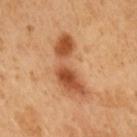Assessment: No biopsy was performed on this lesion — it was imaged during a full skin examination and was not determined to be concerning. Acquisition and patient details: A close-up tile cropped from a whole-body skin photograph, about 15 mm across. The subject is a male aged approximately 50. About 7.5 mm across. The lesion is on the right upper arm. An algorithmic analysis of the crop reported a shape eccentricity near 0.95 and a symmetry-axis asymmetry near 0.45. The software also gave an average lesion color of about L≈52 a*≈26 b*≈38 (CIELAB), roughly 13 lightness units darker than nearby skin, and a lesion-to-skin contrast of about 9 (normalized; higher = more distinct). Captured under cross-polarized illumination.